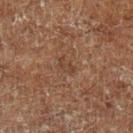size: about 2.5 mm; acquisition: ~15 mm tile from a whole-body skin photo; tile lighting: cross-polarized illumination; patient: male, aged 58 to 62; anatomic site: the left lower leg.